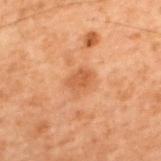No biopsy was performed on this lesion — it was imaged during a full skin examination and was not determined to be concerning.
Located on the upper back.
The subject is a male in their 70s.
Cropped from a total-body skin-imaging series; the visible field is about 15 mm.
Imaged with cross-polarized lighting.
Measured at roughly 2.5 mm in maximum diameter.